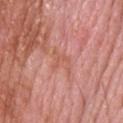Clinical impression:
Recorded during total-body skin imaging; not selected for excision or biopsy.
Image and clinical context:
This image is a 15 mm lesion crop taken from a total-body photograph. The tile uses white-light illumination. A male subject about 65 years old. Longest diameter approximately 3.5 mm. From the upper back.